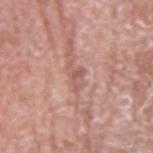This image is a 15 mm lesion crop taken from a total-body photograph. On the right upper arm. About 3.5 mm across. The tile uses white-light illumination. A male subject, in their mid-70s.